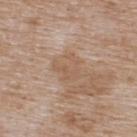biopsy_status: not biopsied; imaged during a skin examination
lighting: white-light
automated_metrics:
  eccentricity: 0.35
  shape_asymmetry: 0.45
  border_irregularity_0_10: 6.5
  color_variation_0_10: 1.5
  peripheral_color_asymmetry: 0.5
  nevus_likeness_0_100: 0
  lesion_detection_confidence_0_100: 100
site: upper back
lesion_size:
  long_diameter_mm_approx: 3.0
patient:
  sex: male
  age_approx: 75
image:
  source: total-body photography crop
  field_of_view_mm: 15